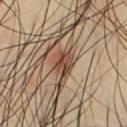follow-up = no biopsy performed (imaged during a skin exam) | body site = the chest | image source = ~15 mm tile from a whole-body skin photo | subject = male, aged 33 to 37.